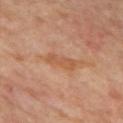  biopsy_status: not biopsied; imaged during a skin examination
  site: mid back
  lighting: cross-polarized
  patient:
    sex: male
    age_approx: 60
  image:
    source: total-body photography crop
    field_of_view_mm: 15